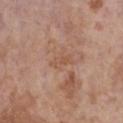| feature | finding |
|---|---|
| workup | catalogued during a skin exam; not biopsied |
| image | ~15 mm tile from a whole-body skin photo |
| lighting | white-light |
| subject | female, aged around 85 or older |
| diameter | ≈3 mm |
| location | the chest |
| image-analysis metrics | a lesion area of about 4 mm², a shape eccentricity near 0.9, and two-axis asymmetry of about 0.25 |